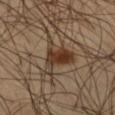Captured during whole-body skin photography for melanoma surveillance; the lesion was not biopsied.
A close-up tile cropped from a whole-body skin photograph, about 15 mm across.
The lesion's longest dimension is about 5.5 mm.
Automated image analysis of the tile measured a lesion area of about 11 mm², an eccentricity of roughly 0.5, and a symmetry-axis asymmetry near 0.6. And it measured an average lesion color of about L≈35 a*≈15 b*≈26 (CIELAB), a lesion–skin lightness drop of about 11, and a normalized lesion–skin contrast near 9.5. The software also gave a border-irregularity index near 7.5/10, internal color variation of about 6.5 on a 0–10 scale, and peripheral color asymmetry of about 2. It also reported lesion-presence confidence of about 100/100.
The subject is a male about 45 years old.
The lesion is located on the right thigh.
The tile uses cross-polarized illumination.